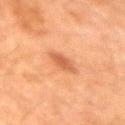Part of a total-body skin-imaging series; this lesion was reviewed on a skin check and was not flagged for biopsy.
The lesion-visualizer software estimated a mean CIELAB color near L≈46 a*≈23 b*≈33, about 8 CIELAB-L* units darker than the surrounding skin, and a lesion-to-skin contrast of about 6.5 (normalized; higher = more distinct).
Captured under cross-polarized illumination.
This image is a 15 mm lesion crop taken from a total-body photograph.
The subject is a male aged approximately 60.
Located on the right upper arm.